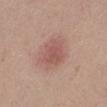Captured during whole-body skin photography for melanoma surveillance; the lesion was not biopsied. A female patient aged approximately 20. Captured under white-light illumination. About 3 mm across. From the left lower leg. A 15 mm close-up extracted from a 3D total-body photography capture.